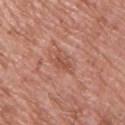Assessment:
The lesion was tiled from a total-body skin photograph and was not biopsied.
Image and clinical context:
About 3.5 mm across. A lesion tile, about 15 mm wide, cut from a 3D total-body photograph. The subject is a male in their mid- to late 60s. The lesion is on the upper back.